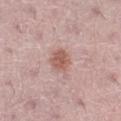Q: Is there a histopathology result?
A: total-body-photography surveillance lesion; no biopsy
Q: How was this image acquired?
A: 15 mm crop, total-body photography
Q: What is the anatomic site?
A: the leg
Q: What is the lesion's diameter?
A: ≈3 mm
Q: Illumination type?
A: white-light
Q: Who is the patient?
A: female, aged approximately 50